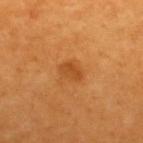Imaged during a routine full-body skin examination; the lesion was not biopsied and no histopathology is available. Automated image analysis of the tile measured an automated nevus-likeness rating near 65 out of 100 and lesion-presence confidence of about 100/100. Imaged with cross-polarized lighting. Approximately 2.5 mm at its widest. The patient is a male in their 60s. On the upper back. A close-up tile cropped from a whole-body skin photograph, about 15 mm across.